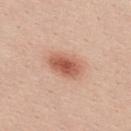biopsy status — imaged on a skin check; not biopsied | lesion diameter — ~4.5 mm (longest diameter) | image — ~15 mm crop, total-body skin-cancer survey | anatomic site — the upper back | patient — female, aged approximately 20.